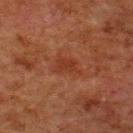Imaged during a routine full-body skin examination; the lesion was not biopsied and no histopathology is available.
The tile uses cross-polarized illumination.
A male patient aged 78 to 82.
A roughly 15 mm field-of-view crop from a total-body skin photograph.
The lesion is on the back.
Longest diameter approximately 3 mm.